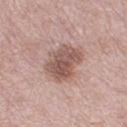notes: catalogued during a skin exam; not biopsied | patient: female, aged 38 to 42 | location: the right thigh | size: ~5.5 mm (longest diameter) | illumination: white-light illumination | image source: ~15 mm tile from a whole-body skin photo.